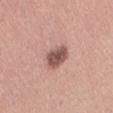Impression:
The lesion was photographed on a routine skin check and not biopsied; there is no pathology result.